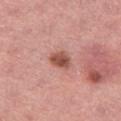Case summary:
• workup · no biopsy performed (imaged during a skin exam)
• subject · female, in their mid- to late 40s
• acquisition · total-body-photography crop, ~15 mm field of view
• tile lighting · white-light
• lesion size · about 3 mm
• anatomic site · the left thigh